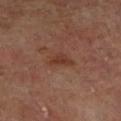  biopsy_status: not biopsied; imaged during a skin examination
  image:
    source: total-body photography crop
    field_of_view_mm: 15
  patient:
    sex: male
    age_approx: 70
  lesion_size:
    long_diameter_mm_approx: 3.0
  site: right lower leg
  automated_metrics:
    area_mm2_approx: 4.0
    eccentricity: 0.75
    shape_asymmetry: 0.4
    border_irregularity_0_10: 4.0
    color_variation_0_10: 1.5
    peripheral_color_asymmetry: 0.5
    lesion_detection_confidence_0_100: 100